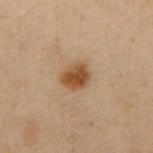No biopsy was performed on this lesion — it was imaged during a full skin examination and was not determined to be concerning.
Approximately 3.5 mm at its widest.
From the abdomen.
The subject is a male aged around 50.
Cropped from a total-body skin-imaging series; the visible field is about 15 mm.
The tile uses cross-polarized illumination.